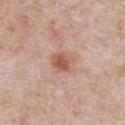notes — no biopsy performed (imaged during a skin exam); imaging modality — total-body-photography crop, ~15 mm field of view; body site — the abdomen; patient — male, about 60 years old.